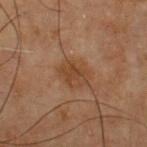Q: Was a biopsy performed?
A: no biopsy performed (imaged during a skin exam)
Q: Lesion size?
A: about 3 mm
Q: Illumination type?
A: cross-polarized
Q: What is the anatomic site?
A: the chest
Q: What kind of image is this?
A: 15 mm crop, total-body photography
Q: Patient demographics?
A: male, aged approximately 70